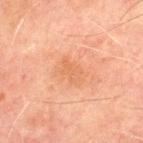- image source: total-body-photography crop, ~15 mm field of view
- patient: male, aged around 70
- tile lighting: cross-polarized illumination
- location: the chest